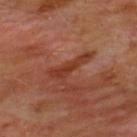Clinical impression:
Part of a total-body skin-imaging series; this lesion was reviewed on a skin check and was not flagged for biopsy.
Clinical summary:
Automated image analysis of the tile measured an eccentricity of roughly 0.95 and a symmetry-axis asymmetry near 0.5. And it measured border irregularity of about 6.5 on a 0–10 scale, internal color variation of about 1.5 on a 0–10 scale, and a peripheral color-asymmetry measure near 0.5. Captured under cross-polarized illumination. The recorded lesion diameter is about 5.5 mm. A male patient approximately 45 years of age. Located on the chest. A 15 mm close-up tile from a total-body photography series done for melanoma screening.